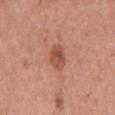Clinical impression: Recorded during total-body skin imaging; not selected for excision or biopsy. Context: From the right upper arm. Imaged with white-light lighting. A region of skin cropped from a whole-body photographic capture, roughly 15 mm wide. The subject is a female in their 50s. An algorithmic analysis of the crop reported an area of roughly 5.5 mm², an outline eccentricity of about 0.7 (0 = round, 1 = elongated), and a symmetry-axis asymmetry near 0.15. The software also gave a nevus-likeness score of about 60/100.